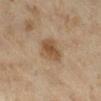Case summary:
- biopsy status — total-body-photography surveillance lesion; no biopsy
- lesion diameter — ≈4 mm
- body site — the right lower leg
- patient — female, aged 38 to 42
- imaging modality — total-body-photography crop, ~15 mm field of view